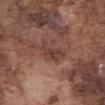Captured during whole-body skin photography for melanoma surveillance; the lesion was not biopsied. This image is a 15 mm lesion crop taken from a total-body photograph. The lesion is located on the abdomen. The patient is a male aged around 75. Longest diameter approximately 3.5 mm. The tile uses white-light illumination.